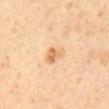Q: Is there a histopathology result?
A: catalogued during a skin exam; not biopsied
Q: How was this image acquired?
A: total-body-photography crop, ~15 mm field of view
Q: What is the anatomic site?
A: the abdomen
Q: Who is the patient?
A: male, in their 60s